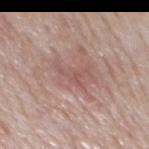Recorded during total-body skin imaging; not selected for excision or biopsy. A close-up tile cropped from a whole-body skin photograph, about 15 mm across. Located on the mid back. Automated tile analysis of the lesion measured roughly 7 lightness units darker than nearby skin. It also reported border irregularity of about 9.5 on a 0–10 scale. The analysis additionally found a nevus-likeness score of about 5/100. The tile uses white-light illumination. The subject is a male aged 73–77.